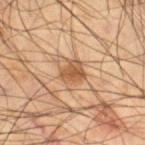Findings:
– notes · imaged on a skin check; not biopsied
– automated lesion analysis · a lesion area of about 6 mm² and a shape-asymmetry score of about 0.3 (0 = symmetric); an average lesion color of about L≈51 a*≈19 b*≈34 (CIELAB), about 11 CIELAB-L* units darker than the surrounding skin, and a lesion-to-skin contrast of about 8 (normalized; higher = more distinct); a border-irregularity rating of about 3/10, a color-variation rating of about 3.5/10, and a peripheral color-asymmetry measure near 1.5; a nevus-likeness score of about 50/100 and a detector confidence of about 100 out of 100 that the crop contains a lesion
– location · the leg
– lighting · cross-polarized illumination
– imaging modality · 15 mm crop, total-body photography
– patient · male, about 55 years old